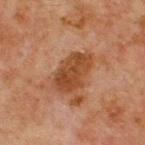| field | value |
|---|---|
| biopsy status | catalogued during a skin exam; not biopsied |
| tile lighting | cross-polarized illumination |
| acquisition | ~15 mm tile from a whole-body skin photo |
| lesion diameter | ~5 mm (longest diameter) |
| patient | male, aged around 65 |
| location | the front of the torso |